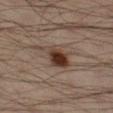workup = imaged on a skin check; not biopsied | location = the right lower leg | illumination = cross-polarized illumination | imaging modality = ~15 mm crop, total-body skin-cancer survey | subject = male, in their mid-30s.